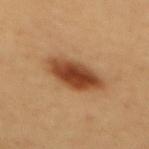Recorded during total-body skin imaging; not selected for excision or biopsy.
This is a cross-polarized tile.
Automated tile analysis of the lesion measured a lesion area of about 14 mm², an outline eccentricity of about 0.85 (0 = round, 1 = elongated), and a shape-asymmetry score of about 0.2 (0 = symmetric). The analysis additionally found a normalized border contrast of about 11.5. The software also gave a detector confidence of about 100 out of 100 that the crop contains a lesion.
The subject is a female approximately 55 years of age.
A 15 mm close-up tile from a total-body photography series done for melanoma screening.
On the mid back.
Approximately 6 mm at its widest.